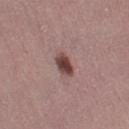image=~15 mm tile from a whole-body skin photo | diameter=about 3 mm | body site=the left thigh | tile lighting=white-light illumination | patient=female, approximately 25 years of age.